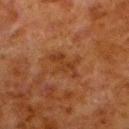diameter: about 4 mm
site: the left lower leg
automated lesion analysis: a footprint of about 5.5 mm², an outline eccentricity of about 0.9 (0 = round, 1 = elongated), and a symmetry-axis asymmetry near 0.5; an average lesion color of about L≈30 a*≈20 b*≈30 (CIELAB), about 6 CIELAB-L* units darker than the surrounding skin, and a normalized border contrast of about 6; a border-irregularity rating of about 7/10, a color-variation rating of about 2/10, and a peripheral color-asymmetry measure near 0.5; an automated nevus-likeness rating near 0 out of 100 and a lesion-detection confidence of about 100/100
imaging modality: total-body-photography crop, ~15 mm field of view
patient: male, about 80 years old
illumination: cross-polarized